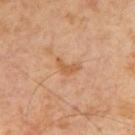Assessment: The lesion was tiled from a total-body skin photograph and was not biopsied. Background: Approximately 3 mm at its widest. This image is a 15 mm lesion crop taken from a total-body photograph. The total-body-photography lesion software estimated an average lesion color of about L≈56 a*≈22 b*≈37 (CIELAB), roughly 8 lightness units darker than nearby skin, and a normalized lesion–skin contrast near 6.5. Captured under cross-polarized illumination. The subject is a male aged 63 to 67.